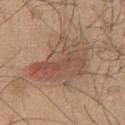| field | value |
|---|---|
| biopsy status | total-body-photography surveillance lesion; no biopsy |
| automated metrics | about 9 CIELAB-L* units darker than the surrounding skin and a normalized border contrast of about 7; a border-irregularity rating of about 5.5/10 and a color-variation rating of about 7.5/10; a nevus-likeness score of about 5/100 and a detector confidence of about 95 out of 100 that the crop contains a lesion |
| tile lighting | white-light |
| location | the chest |
| patient | male, approximately 45 years of age |
| acquisition | ~15 mm crop, total-body skin-cancer survey |
| size | about 9 mm |